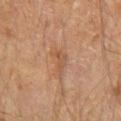No biopsy was performed on this lesion — it was imaged during a full skin examination and was not determined to be concerning. The lesion is located on the right upper arm. A 15 mm close-up extracted from a 3D total-body photography capture. Captured under cross-polarized illumination. A male subject, in their mid-40s.